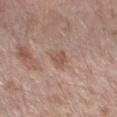Q: Was this lesion biopsied?
A: catalogued during a skin exam; not biopsied
Q: Lesion size?
A: ~3 mm (longest diameter)
Q: What kind of image is this?
A: ~15 mm tile from a whole-body skin photo
Q: Who is the patient?
A: male, approximately 70 years of age
Q: Where on the body is the lesion?
A: the right forearm